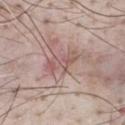| feature | finding |
|---|---|
| notes | catalogued during a skin exam; not biopsied |
| image | total-body-photography crop, ~15 mm field of view |
| automated metrics | a lesion area of about 6.5 mm², a shape eccentricity near 0.9, and two-axis asymmetry of about 0.4; a border-irregularity rating of about 5.5/10, internal color variation of about 4 on a 0–10 scale, and peripheral color asymmetry of about 1.5; a classifier nevus-likeness of about 0/100 |
| tile lighting | white-light illumination |
| subject | male, aged 53 to 57 |